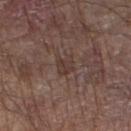workup = total-body-photography surveillance lesion; no biopsy | TBP lesion metrics = a footprint of about 4.5 mm² and two-axis asymmetry of about 0.25; a border-irregularity rating of about 2.5/10, a color-variation rating of about 2/10, and a peripheral color-asymmetry measure near 1; a nevus-likeness score of about 0/100 | image source = total-body-photography crop, ~15 mm field of view | tile lighting = white-light | location = the left lower leg | subject = male, in their 80s.